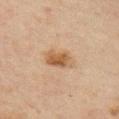Notes:
- image · ~15 mm crop, total-body skin-cancer survey
- tile lighting · cross-polarized
- image-analysis metrics · a lesion area of about 7.5 mm², an outline eccentricity of about 0.8 (0 = round, 1 = elongated), and a symmetry-axis asymmetry near 0.15; a lesion color around L≈49 a*≈17 b*≈32 in CIELAB, roughly 9 lightness units darker than nearby skin, and a normalized lesion–skin contrast near 8; a nevus-likeness score of about 85/100
- location · the right upper arm
- patient · male, in their mid-40s